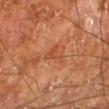Captured during whole-body skin photography for melanoma surveillance; the lesion was not biopsied.
The subject is a male about 65 years old.
From the left lower leg.
A lesion tile, about 15 mm wide, cut from a 3D total-body photograph.